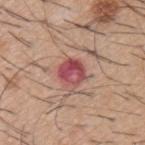follow-up: catalogued during a skin exam; not biopsied | body site: the back | subject: male, aged around 60 | lighting: white-light illumination | image source: ~15 mm crop, total-body skin-cancer survey | automated metrics: a mean CIELAB color near L≈49 a*≈32 b*≈21, a lesion–skin lightness drop of about 13, and a normalized border contrast of about 10; a within-lesion color-variation index near 7.5/10 and radial color variation of about 3.